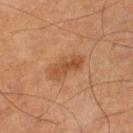Imaged during a routine full-body skin examination; the lesion was not biopsied and no histopathology is available.
From the right lower leg.
The total-body-photography lesion software estimated a footprint of about 7 mm², a shape eccentricity near 0.9, and a symmetry-axis asymmetry near 0.3.
The tile uses cross-polarized illumination.
A male patient, in their mid- to late 60s.
A close-up tile cropped from a whole-body skin photograph, about 15 mm across.
About 4.5 mm across.